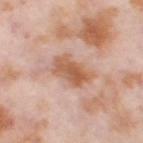The lesion was tiled from a total-body skin photograph and was not biopsied. The tile uses cross-polarized illumination. From the right thigh. Measured at roughly 4.5 mm in maximum diameter. A region of skin cropped from a whole-body photographic capture, roughly 15 mm wide. A female subject in their mid-50s.